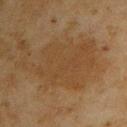Captured during whole-body skin photography for melanoma surveillance; the lesion was not biopsied.
The recorded lesion diameter is about 10 mm.
This is a cross-polarized tile.
Cropped from a whole-body photographic skin survey; the tile spans about 15 mm.
An algorithmic analysis of the crop reported a lesion color around L≈35 a*≈14 b*≈29 in CIELAB, roughly 5 lightness units darker than nearby skin, and a lesion-to-skin contrast of about 5 (normalized; higher = more distinct). The software also gave a border-irregularity index near 7.5/10, internal color variation of about 2.5 on a 0–10 scale, and a peripheral color-asymmetry measure near 1. It also reported an automated nevus-likeness rating near 0 out of 100.
From the right upper arm.
The subject is a male in their mid-40s.